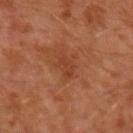biopsy_status: not biopsied; imaged during a skin examination
patient:
  sex: male
  age_approx: 30
site: left forearm
lesion_size:
  long_diameter_mm_approx: 2.5
image:
  source: total-body photography crop
  field_of_view_mm: 15
lighting: cross-polarized